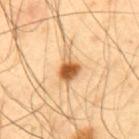{
  "biopsy_status": "not biopsied; imaged during a skin examination",
  "patient": {
    "sex": "male",
    "age_approx": 40
  },
  "image": {
    "source": "total-body photography crop",
    "field_of_view_mm": 15
  },
  "lighting": "cross-polarized",
  "site": "upper back"
}